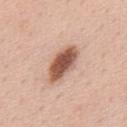Clinical impression: This lesion was catalogued during total-body skin photography and was not selected for biopsy. Image and clinical context: A male patient about 60 years old. Imaged with white-light lighting. A 15 mm crop from a total-body photograph taken for skin-cancer surveillance. The lesion is on the upper back.